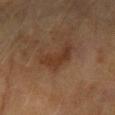| field | value |
|---|---|
| workup | total-body-photography surveillance lesion; no biopsy |
| image source | total-body-photography crop, ~15 mm field of view |
| lesion diameter | about 4.5 mm |
| subject | female, aged 58 to 62 |
| anatomic site | the left forearm |